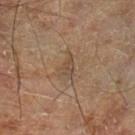The lesion was photographed on a routine skin check and not biopsied; there is no pathology result. The total-body-photography lesion software estimated a classifier nevus-likeness of about 0/100 and a detector confidence of about 95 out of 100 that the crop contains a lesion. A 15 mm close-up extracted from a 3D total-body photography capture. Imaged with cross-polarized lighting. Located on the leg. The recorded lesion diameter is about 2.5 mm. A male subject, aged 68 to 72.